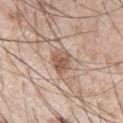Q: Was a biopsy performed?
A: total-body-photography surveillance lesion; no biopsy
Q: What is the anatomic site?
A: the arm
Q: What lighting was used for the tile?
A: white-light
Q: What kind of image is this?
A: total-body-photography crop, ~15 mm field of view
Q: How large is the lesion?
A: about 3.5 mm
Q: Who is the patient?
A: male, approximately 55 years of age